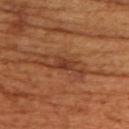Background: A 15 mm close-up extracted from a 3D total-body photography capture. Longest diameter approximately 3 mm. The subject is a female roughly 55 years of age. Automated tile analysis of the lesion measured a mean CIELAB color near L≈39 a*≈25 b*≈34, roughly 9 lightness units darker than nearby skin, and a normalized border contrast of about 7.5. The analysis additionally found internal color variation of about 2.5 on a 0–10 scale and peripheral color asymmetry of about 1. The lesion is on the upper back.